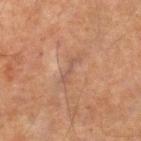The lesion was tiled from a total-body skin photograph and was not biopsied.
The lesion is on the arm.
A roughly 15 mm field-of-view crop from a total-body skin photograph.
The subject is a male aged 63 to 67.